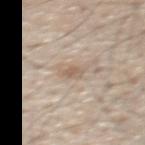The lesion was photographed on a routine skin check and not biopsied; there is no pathology result. This is a white-light tile. The lesion's longest dimension is about 3.5 mm. The subject is a male aged 58 to 62. A 15 mm crop from a total-body photograph taken for skin-cancer surveillance. From the upper back.